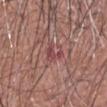Clinical impression:
The lesion was photographed on a routine skin check and not biopsied; there is no pathology result.
Clinical summary:
Automated tile analysis of the lesion measured a mean CIELAB color near L≈46 a*≈28 b*≈20, roughly 8 lightness units darker than nearby skin, and a normalized lesion–skin contrast near 6.5. The software also gave a border-irregularity index near 7.5/10, a color-variation rating of about 1.5/10, and peripheral color asymmetry of about 0.5. Approximately 3 mm at its widest. A 15 mm crop from a total-body photograph taken for skin-cancer surveillance. Imaged with white-light lighting. The lesion is located on the head or neck. A male subject, aged approximately 65.